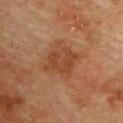Findings:
- notes — total-body-photography surveillance lesion; no biopsy
- imaging modality — total-body-photography crop, ~15 mm field of view
- body site — the chest
- image-analysis metrics — a footprint of about 9.5 mm², an outline eccentricity of about 0.55 (0 = round, 1 = elongated), and a symmetry-axis asymmetry near 0.3; an average lesion color of about L≈36 a*≈21 b*≈29 (CIELAB), roughly 7 lightness units darker than nearby skin, and a normalized lesion–skin contrast near 6; a border-irregularity rating of about 3.5/10 and internal color variation of about 2.5 on a 0–10 scale
- size — ≈4 mm
- tile lighting — cross-polarized illumination
- subject — male, roughly 75 years of age